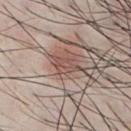Imaged during a routine full-body skin examination; the lesion was not biopsied and no histopathology is available. A male patient, aged around 25. Automated image analysis of the tile measured an area of roughly 4.5 mm², a shape eccentricity near 0.8, and a shape-asymmetry score of about 0.4 (0 = symmetric). And it measured an average lesion color of about L≈51 a*≈19 b*≈23 (CIELAB), a lesion–skin lightness drop of about 8, and a normalized lesion–skin contrast near 6.5. The lesion is located on the chest. A roughly 15 mm field-of-view crop from a total-body skin photograph. The tile uses white-light illumination.